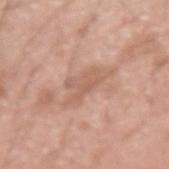Q: Was this lesion biopsied?
A: imaged on a skin check; not biopsied
Q: Who is the patient?
A: male, aged approximately 20
Q: Lesion location?
A: the arm
Q: What kind of image is this?
A: total-body-photography crop, ~15 mm field of view
Q: How large is the lesion?
A: ≈3.5 mm